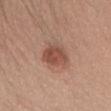biopsy status: catalogued during a skin exam; not biopsied | automated metrics: an area of roughly 8.5 mm² and two-axis asymmetry of about 0.2; a nevus-likeness score of about 95/100 and a detector confidence of about 100 out of 100 that the crop contains a lesion | size: ~3.5 mm (longest diameter) | image: 15 mm crop, total-body photography | subject: female, aged around 35 | body site: the head or neck.